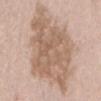The lesion was photographed on a routine skin check and not biopsied; there is no pathology result.
The total-body-photography lesion software estimated a border-irregularity rating of about 4.5/10 and a color-variation rating of about 4/10.
The recorded lesion diameter is about 11.5 mm.
The tile uses white-light illumination.
Located on the lower back.
A close-up tile cropped from a whole-body skin photograph, about 15 mm across.
A male subject, aged 38–42.